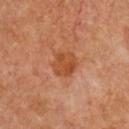{"biopsy_status": "not biopsied; imaged during a skin examination", "lighting": "cross-polarized", "patient": {"sex": "female", "age_approx": 65}, "image": {"source": "total-body photography crop", "field_of_view_mm": 15}, "automated_metrics": {"area_mm2_approx": 7.5, "eccentricity": 0.35, "shape_asymmetry": 0.2, "cielab_L": 48, "cielab_a": 27, "cielab_b": 38}, "site": "chest"}